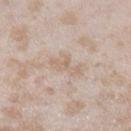Impression: The lesion was photographed on a routine skin check and not biopsied; there is no pathology result. Background: Captured under white-light illumination. On the left lower leg. Cropped from a whole-body photographic skin survey; the tile spans about 15 mm. A female subject aged 23–27. Automated tile analysis of the lesion measured a lesion area of about 5.5 mm², an eccentricity of roughly 0.9, and a shape-asymmetry score of about 0.45 (0 = symmetric).